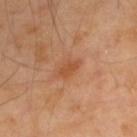Findings:
- notes: imaged on a skin check; not biopsied
- size: ~3 mm (longest diameter)
- illumination: cross-polarized
- body site: the back
- imaging modality: total-body-photography crop, ~15 mm field of view
- automated lesion analysis: a mean CIELAB color near L≈51 a*≈26 b*≈37, about 8 CIELAB-L* units darker than the surrounding skin, and a lesion-to-skin contrast of about 6.5 (normalized; higher = more distinct); border irregularity of about 3 on a 0–10 scale and internal color variation of about 1 on a 0–10 scale; an automated nevus-likeness rating near 10 out of 100 and a detector confidence of about 100 out of 100 that the crop contains a lesion
- patient: male, aged 38 to 42